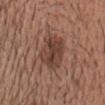| field | value |
|---|---|
| automated lesion analysis | a footprint of about 14 mm², a shape eccentricity near 0.7, and a shape-asymmetry score of about 0.2 (0 = symmetric); an average lesion color of about L≈41 a*≈20 b*≈25 (CIELAB) and a normalized lesion–skin contrast near 8; border irregularity of about 2 on a 0–10 scale, a color-variation rating of about 5/10, and radial color variation of about 2; an automated nevus-likeness rating near 35 out of 100 |
| anatomic site | the head or neck |
| lighting | white-light |
| acquisition | ~15 mm tile from a whole-body skin photo |
| patient | male, aged 38–42 |